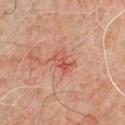A male patient, approximately 50 years of age.
A 15 mm close-up tile from a total-body photography series done for melanoma screening.
The lesion is located on the chest.